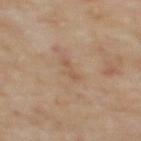Findings:
• workup — no biopsy performed (imaged during a skin exam)
• subject — female, aged 58 to 62
• image source — ~15 mm tile from a whole-body skin photo
• lesion diameter — about 2.5 mm
• location — the upper back
• tile lighting — cross-polarized illumination